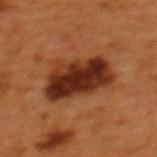Case summary:
- image source: ~15 mm tile from a whole-body skin photo
- lighting: cross-polarized illumination
- location: the back
- lesion diameter: ~6.5 mm (longest diameter)
- subject: female, about 50 years old
- automated lesion analysis: a footprint of about 22 mm², an outline eccentricity of about 0.8 (0 = round, 1 = elongated), and two-axis asymmetry of about 0.2; a lesion-detection confidence of about 100/100